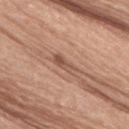* workup — catalogued during a skin exam; not biopsied
* image source — ~15 mm crop, total-body skin-cancer survey
* subject — male, aged approximately 70
* tile lighting — white-light illumination
* anatomic site — the lower back